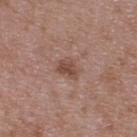follow-up: total-body-photography surveillance lesion; no biopsy
patient: male, aged 48–52
automated metrics: a lesion area of about 5 mm², an outline eccentricity of about 0.65 (0 = round, 1 = elongated), and a symmetry-axis asymmetry near 0.3; an average lesion color of about L≈48 a*≈20 b*≈26 (CIELAB) and a lesion–skin lightness drop of about 9; an automated nevus-likeness rating near 50 out of 100 and a detector confidence of about 100 out of 100 that the crop contains a lesion
acquisition: ~15 mm crop, total-body skin-cancer survey
anatomic site: the upper back
diameter: about 3 mm
tile lighting: white-light